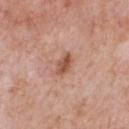No biopsy was performed on this lesion — it was imaged during a full skin examination and was not determined to be concerning. A male subject, in their 60s. A 15 mm close-up tile from a total-body photography series done for melanoma screening. On the chest. Imaged with white-light lighting. Automated image analysis of the tile measured a footprint of about 4 mm², an eccentricity of roughly 0.8, and a shape-asymmetry score of about 0.25 (0 = symmetric). The software also gave an automated nevus-likeness rating near 75 out of 100. Longest diameter approximately 3 mm.